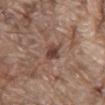| key | value |
|---|---|
| workup | no biopsy performed (imaged during a skin exam) |
| diameter | about 3 mm |
| image-analysis metrics | a lesion color around L≈42 a*≈18 b*≈24 in CIELAB and a normalized lesion–skin contrast near 8.5; border irregularity of about 3 on a 0–10 scale, a within-lesion color-variation index near 2/10, and peripheral color asymmetry of about 0.5 |
| subject | male, roughly 80 years of age |
| image | ~15 mm tile from a whole-body skin photo |
| tile lighting | white-light illumination |
| anatomic site | the mid back |